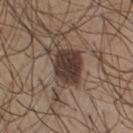Notes:
– acquisition · 15 mm crop, total-body photography
– body site · the front of the torso
– patient · male, in their mid- to late 20s